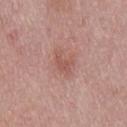workup = total-body-photography surveillance lesion; no biopsy
lesion size = ~3 mm (longest diameter)
lighting = white-light
patient = male, roughly 30 years of age
acquisition = 15 mm crop, total-body photography